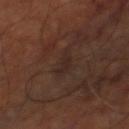{"biopsy_status": "not biopsied; imaged during a skin examination", "image": {"source": "total-body photography crop", "field_of_view_mm": 15}, "lesion_size": {"long_diameter_mm_approx": 3.5}, "patient": {"age_approx": 65}, "site": "right thigh", "automated_metrics": {"vs_skin_darker_L": 5.0, "vs_skin_contrast_norm": 6.0, "peripheral_color_asymmetry": 0.5, "nevus_likeness_0_100": 0}, "lighting": "cross-polarized"}